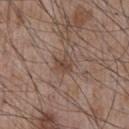A region of skin cropped from a whole-body photographic capture, roughly 15 mm wide. Located on the front of the torso. This is a white-light tile. The recorded lesion diameter is about 2.5 mm. A male subject, approximately 55 years of age.